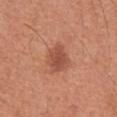The lesion was tiled from a total-body skin photograph and was not biopsied. From the abdomen. This is a white-light tile. An algorithmic analysis of the crop reported about 10 CIELAB-L* units darker than the surrounding skin and a normalized border contrast of about 7. Longest diameter approximately 3 mm. A 15 mm close-up tile from a total-body photography series done for melanoma screening. A male patient in their mid-60s.